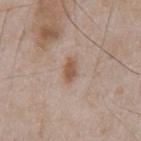Notes:
– notes · total-body-photography surveillance lesion; no biopsy
– site · the chest
– patient · male, aged 63–67
– image · ~15 mm tile from a whole-body skin photo